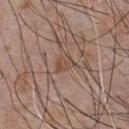Part of a total-body skin-imaging series; this lesion was reviewed on a skin check and was not flagged for biopsy.